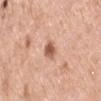The lesion was tiled from a total-body skin photograph and was not biopsied. A male patient approximately 40 years of age. About 2.5 mm across. Cropped from a whole-body photographic skin survey; the tile spans about 15 mm. From the left upper arm. The lesion-visualizer software estimated a border-irregularity rating of about 2/10, a within-lesion color-variation index near 3/10, and peripheral color asymmetry of about 1. The software also gave an automated nevus-likeness rating near 90 out of 100 and lesion-presence confidence of about 100/100.